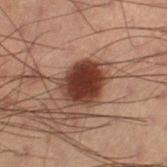Q: Was a biopsy performed?
A: catalogued during a skin exam; not biopsied
Q: What is the lesion's diameter?
A: ≈4.5 mm
Q: How was the tile lit?
A: cross-polarized illumination
Q: Lesion location?
A: the leg
Q: Patient demographics?
A: male, aged 53–57
Q: What is the imaging modality?
A: ~15 mm tile from a whole-body skin photo